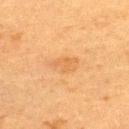Clinical impression:
Part of a total-body skin-imaging series; this lesion was reviewed on a skin check and was not flagged for biopsy.
Image and clinical context:
A female patient, aged around 55. A 15 mm crop from a total-body photograph taken for skin-cancer surveillance. Located on the upper back.